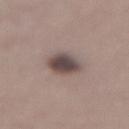This lesion was catalogued during total-body skin photography and was not selected for biopsy.
Imaged with white-light lighting.
A female subject, approximately 45 years of age.
On the lower back.
Approximately 4 mm at its widest.
A 15 mm close-up tile from a total-body photography series done for melanoma screening.